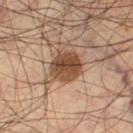Assessment:
The lesion was tiled from a total-body skin photograph and was not biopsied.
Context:
Cropped from a whole-body photographic skin survey; the tile spans about 15 mm. A male subject, aged approximately 55. The lesion-visualizer software estimated an area of roughly 11 mm², an outline eccentricity of about 0.55 (0 = round, 1 = elongated), and a symmetry-axis asymmetry near 0.15. And it measured an average lesion color of about L≈43 a*≈18 b*≈28 (CIELAB), a lesion–skin lightness drop of about 13, and a lesion-to-skin contrast of about 10.5 (normalized; higher = more distinct). It also reported border irregularity of about 1.5 on a 0–10 scale and a color-variation rating of about 4.5/10. The recorded lesion diameter is about 4 mm. The lesion is located on the left thigh.